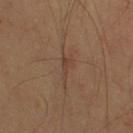The lesion was photographed on a routine skin check and not biopsied; there is no pathology result.
A region of skin cropped from a whole-body photographic capture, roughly 15 mm wide.
An algorithmic analysis of the crop reported border irregularity of about 6 on a 0–10 scale, a within-lesion color-variation index near 0/10, and radial color variation of about 0. The analysis additionally found a detector confidence of about 75 out of 100 that the crop contains a lesion.
A male subject roughly 70 years of age.
From the upper back.
Imaged with cross-polarized lighting.